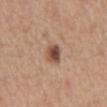Impression:
Recorded during total-body skin imaging; not selected for excision or biopsy.
Image and clinical context:
The lesion is on the abdomen. The patient is a male aged 63 to 67. A 15 mm close-up tile from a total-body photography series done for melanoma screening.